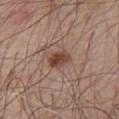workup: no biopsy performed (imaged during a skin exam) | location: the chest | tile lighting: white-light | subject: male, roughly 65 years of age | image source: ~15 mm tile from a whole-body skin photo | size: ~3 mm (longest diameter).